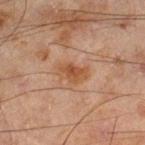acquisition — ~15 mm crop, total-body skin-cancer survey
patient — male, in their mid- to late 40s
site — the right lower leg
lighting — cross-polarized illumination